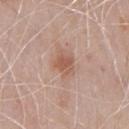  biopsy_status: not biopsied; imaged during a skin examination
  image:
    source: total-body photography crop
    field_of_view_mm: 15
  lesion_size:
    long_diameter_mm_approx: 2.5
  site: front of the torso
  automated_metrics:
    area_mm2_approx: 4.5
    eccentricity: 0.5
    vs_skin_darker_L: 9.0
    vs_skin_contrast_norm: 7.0
  patient:
    sex: male
    age_approx: 75
  lighting: white-light